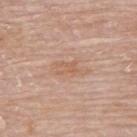The lesion was photographed on a routine skin check and not biopsied; there is no pathology result. A female patient aged approximately 65. A 15 mm close-up extracted from a 3D total-body photography capture. The recorded lesion diameter is about 4 mm. The tile uses white-light illumination. From the upper back.